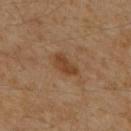The lesion was tiled from a total-body skin photograph and was not biopsied. About 3.5 mm across. The tile uses cross-polarized illumination. A 15 mm close-up extracted from a 3D total-body photography capture. The lesion is located on the upper back. The patient is a male in their 60s.